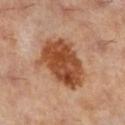Part of a total-body skin-imaging series; this lesion was reviewed on a skin check and was not flagged for biopsy. A roughly 15 mm field-of-view crop from a total-body skin photograph. The lesion is located on the right lower leg. The patient is a female roughly 60 years of age.